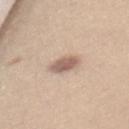Case summary:
* biopsy status: imaged on a skin check; not biopsied
* patient: female, in their mid- to late 30s
* acquisition: 15 mm crop, total-body photography
* tile lighting: white-light
* anatomic site: the mid back
* diameter: about 3 mm